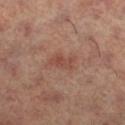{"biopsy_status": "not biopsied; imaged during a skin examination", "lesion_size": {"long_diameter_mm_approx": 3.0}, "image": {"source": "total-body photography crop", "field_of_view_mm": 15}, "automated_metrics": {"shape_asymmetry": 0.35, "cielab_L": 48, "cielab_a": 24, "cielab_b": 28, "vs_skin_darker_L": 7.0, "vs_skin_contrast_norm": 6.0, "lesion_detection_confidence_0_100": 100}, "site": "leg", "patient": {"sex": "female", "age_approx": 60}, "lighting": "cross-polarized"}